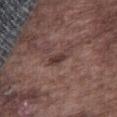The lesion was photographed on a routine skin check and not biopsied; there is no pathology result. An algorithmic analysis of the crop reported a normalized border contrast of about 7.5. The software also gave a within-lesion color-variation index near 2.5/10 and a peripheral color-asymmetry measure near 1. The tile uses white-light illumination. The subject is a male in their mid- to late 70s. This image is a 15 mm lesion crop taken from a total-body photograph. On the front of the torso.